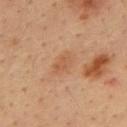Impression: The lesion was tiled from a total-body skin photograph and was not biopsied. Context: A 15 mm close-up extracted from a 3D total-body photography capture. A male subject, roughly 35 years of age. The lesion is located on the upper back. Captured under cross-polarized illumination. The total-body-photography lesion software estimated a lesion area of about 6 mm² and an outline eccentricity of about 0.8 (0 = round, 1 = elongated). The analysis additionally found a lesion color around L≈51 a*≈20 b*≈32 in CIELAB and a lesion–skin lightness drop of about 6. The analysis additionally found a classifier nevus-likeness of about 0/100 and a detector confidence of about 100 out of 100 that the crop contains a lesion.